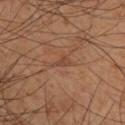Image and clinical context: Captured under cross-polarized illumination. Located on the upper back. The patient is a male aged around 50. A 15 mm crop from a total-body photograph taken for skin-cancer surveillance. Measured at roughly 2.5 mm in maximum diameter. Automated tile analysis of the lesion measured a lesion area of about 3 mm², an outline eccentricity of about 0.85 (0 = round, 1 = elongated), and a shape-asymmetry score of about 0.6 (0 = symmetric). And it measured a mean CIELAB color near L≈42 a*≈20 b*≈28, roughly 6 lightness units darker than nearby skin, and a lesion-to-skin contrast of about 5 (normalized; higher = more distinct). The analysis additionally found a color-variation rating of about 0/10 and radial color variation of about 0.